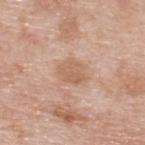The lesion was photographed on a routine skin check and not biopsied; there is no pathology result. Measured at roughly 3.5 mm in maximum diameter. A male subject aged 58–62. A close-up tile cropped from a whole-body skin photograph, about 15 mm across. Located on the upper back.